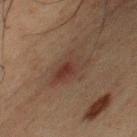Clinical impression:
No biopsy was performed on this lesion — it was imaged during a full skin examination and was not determined to be concerning.
Clinical summary:
The subject is a male in their 50s. On the chest. A roughly 15 mm field-of-view crop from a total-body skin photograph.